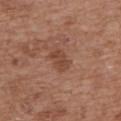The lesion was photographed on a routine skin check and not biopsied; there is no pathology result.
A female patient, approximately 75 years of age.
The lesion is on the upper back.
Automated image analysis of the tile measured a mean CIELAB color near L≈45 a*≈22 b*≈29, a lesion–skin lightness drop of about 8, and a normalized lesion–skin contrast near 6.5. The software also gave an automated nevus-likeness rating near 60 out of 100.
Approximately 3 mm at its widest.
Cropped from a total-body skin-imaging series; the visible field is about 15 mm.